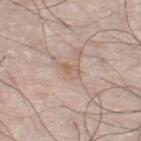The lesion was photographed on a routine skin check and not biopsied; there is no pathology result. A male subject, aged 68–72. The lesion's longest dimension is about 2.5 mm. A region of skin cropped from a whole-body photographic capture, roughly 15 mm wide. On the left leg. The tile uses white-light illumination.